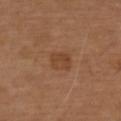Q: Is there a histopathology result?
A: catalogued during a skin exam; not biopsied
Q: What are the patient's age and sex?
A: male, aged 73 to 77
Q: Lesion location?
A: the upper back
Q: How was this image acquired?
A: 15 mm crop, total-body photography